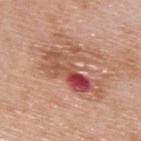biopsy status — catalogued during a skin exam; not biopsied
subject — male, about 80 years old
acquisition — 15 mm crop, total-body photography
tile lighting — white-light illumination
anatomic site — the upper back
automated metrics — a lesion area of about 20 mm², a shape eccentricity near 0.85, and two-axis asymmetry of about 0.5; a lesion color around L≈54 a*≈26 b*≈30 in CIELAB and roughly 11 lightness units darker than nearby skin